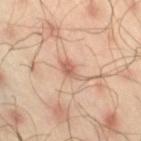The lesion was photographed on a routine skin check and not biopsied; there is no pathology result.
The recorded lesion diameter is about 4 mm.
The lesion is located on the right thigh.
The total-body-photography lesion software estimated a border-irregularity rating of about 4.5/10, internal color variation of about 1.5 on a 0–10 scale, and a peripheral color-asymmetry measure near 0.5. And it measured an automated nevus-likeness rating near 10 out of 100 and lesion-presence confidence of about 100/100.
A male subject in their mid- to late 40s.
This is a cross-polarized tile.
A lesion tile, about 15 mm wide, cut from a 3D total-body photograph.